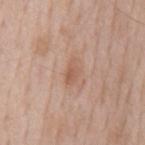Impression:
Part of a total-body skin-imaging series; this lesion was reviewed on a skin check and was not flagged for biopsy.
Image and clinical context:
A roughly 15 mm field-of-view crop from a total-body skin photograph. The lesion is located on the mid back. A male subject, aged around 60.